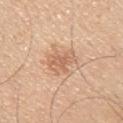{"biopsy_status": "not biopsied; imaged during a skin examination", "site": "right upper arm", "lesion_size": {"long_diameter_mm_approx": 3.5}, "image": {"source": "total-body photography crop", "field_of_view_mm": 15}, "patient": {"sex": "male", "age_approx": 45}, "lighting": "white-light", "automated_metrics": {"eccentricity": 0.4, "shape_asymmetry": 0.3, "peripheral_color_asymmetry": 1.0}}